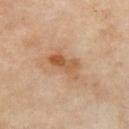notes — catalogued during a skin exam; not biopsied
subject — female, roughly 50 years of age
anatomic site — the chest
image — ~15 mm tile from a whole-body skin photo
illumination — cross-polarized
automated metrics — a footprint of about 8 mm², an eccentricity of roughly 0.85, and a shape-asymmetry score of about 0.3 (0 = symmetric); a lesion color around L≈55 a*≈20 b*≈35 in CIELAB, a lesion–skin lightness drop of about 9, and a lesion-to-skin contrast of about 7.5 (normalized; higher = more distinct)
diameter — about 4.5 mm